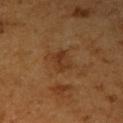The lesion was tiled from a total-body skin photograph and was not biopsied.
Automated tile analysis of the lesion measured a lesion color around L≈35 a*≈20 b*≈33 in CIELAB and about 6 CIELAB-L* units darker than the surrounding skin.
This image is a 15 mm lesion crop taken from a total-body photograph.
The lesion is located on the right upper arm.
Measured at roughly 3 mm in maximum diameter.
The subject is a male aged around 60.
This is a cross-polarized tile.